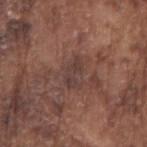<lesion>
  <biopsy_status>not biopsied; imaged during a skin examination</biopsy_status>
  <automated_metrics>
    <area_mm2_approx>5.0</area_mm2_approx>
    <shape_asymmetry>0.4</shape_asymmetry>
    <color_variation_0_10>2.5</color_variation_0_10>
    <peripheral_color_asymmetry>1.0</peripheral_color_asymmetry>
  </automated_metrics>
  <lighting>white-light</lighting>
  <patient>
    <sex>male</sex>
    <age_approx>75</age_approx>
  </patient>
  <site>right upper arm</site>
  <image>
    <source>total-body photography crop</source>
    <field_of_view_mm>15</field_of_view_mm>
  </image>
</lesion>